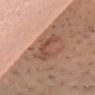{"biopsy_status": "not biopsied; imaged during a skin examination", "site": "front of the torso", "patient": {"sex": "female", "age_approx": 45}, "image": {"source": "total-body photography crop", "field_of_view_mm": 15}, "lighting": "white-light"}